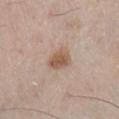biopsy_status: not biopsied; imaged during a skin examination
patient:
  sex: male
  age_approx: 60
site: right lower leg
image:
  source: total-body photography crop
  field_of_view_mm: 15